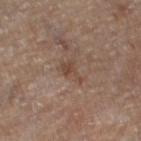Clinical impression:
The lesion was photographed on a routine skin check and not biopsied; there is no pathology result.
Background:
Automated tile analysis of the lesion measured a border-irregularity index near 6/10 and internal color variation of about 1 on a 0–10 scale. A male subject, roughly 85 years of age or older. Cropped from a total-body skin-imaging series; the visible field is about 15 mm. The lesion is on the leg. The tile uses cross-polarized illumination. About 3 mm across.